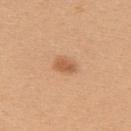The patient is a female aged 23–27.
The recorded lesion diameter is about 2.5 mm.
Located on the upper back.
An algorithmic analysis of the crop reported a shape eccentricity near 0.75. It also reported an average lesion color of about L≈59 a*≈23 b*≈37 (CIELAB), about 11 CIELAB-L* units darker than the surrounding skin, and a lesion-to-skin contrast of about 7 (normalized; higher = more distinct). The analysis additionally found border irregularity of about 2 on a 0–10 scale, internal color variation of about 1.5 on a 0–10 scale, and a peripheral color-asymmetry measure near 0.5. The analysis additionally found a classifier nevus-likeness of about 95/100.
A roughly 15 mm field-of-view crop from a total-body skin photograph.